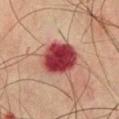follow-up = total-body-photography surveillance lesion; no biopsy
subject = male, aged around 75
anatomic site = the chest
lesion diameter = ≈5 mm
TBP lesion metrics = border irregularity of about 1.5 on a 0–10 scale and a within-lesion color-variation index near 6.5/10
image = total-body-photography crop, ~15 mm field of view
tile lighting = cross-polarized illumination